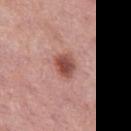| key | value |
|---|---|
| workup | catalogued during a skin exam; not biopsied |
| image | total-body-photography crop, ~15 mm field of view |
| lesion diameter | ~3 mm (longest diameter) |
| lighting | white-light |
| subject | female, approximately 50 years of age |
| anatomic site | the left thigh |